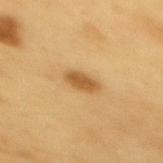- follow-up — no biopsy performed (imaged during a skin exam)
- location — the upper back
- diameter — ~3.5 mm (longest diameter)
- image — ~15 mm tile from a whole-body skin photo
- TBP lesion metrics — a lesion area of about 5 mm², a shape eccentricity near 0.85, and a shape-asymmetry score of about 0.2 (0 = symmetric); a mean CIELAB color near L≈56 a*≈20 b*≈43, roughly 12 lightness units darker than nearby skin, and a lesion-to-skin contrast of about 8 (normalized; higher = more distinct); border irregularity of about 2 on a 0–10 scale and a within-lesion color-variation index near 2/10
- patient — male, in their 60s